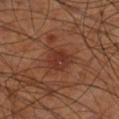Assessment: The lesion was photographed on a routine skin check and not biopsied; there is no pathology result. Clinical summary: A male patient roughly 70 years of age. The lesion is on the right lower leg. A 15 mm close-up extracted from a 3D total-body photography capture. Automated image analysis of the tile measured an area of roughly 8 mm², an eccentricity of roughly 0.55, and a symmetry-axis asymmetry near 0.25. The analysis additionally found an average lesion color of about L≈32 a*≈23 b*≈27 (CIELAB), a lesion–skin lightness drop of about 7, and a normalized border contrast of about 6.5. About 3.5 mm across.